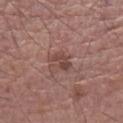workup: catalogued during a skin exam; not biopsied | illumination: white-light | TBP lesion metrics: a footprint of about 4 mm²; a lesion color around L≈44 a*≈21 b*≈23 in CIELAB and a normalized border contrast of about 6.5; a border-irregularity index near 3.5/10, internal color variation of about 2 on a 0–10 scale, and a peripheral color-asymmetry measure near 0.5; an automated nevus-likeness rating near 0 out of 100 and lesion-presence confidence of about 100/100 | acquisition: ~15 mm tile from a whole-body skin photo | size: ~3 mm (longest diameter) | subject: male, about 65 years old | location: the leg.